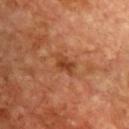biopsy status — total-body-photography surveillance lesion; no biopsy
site — the chest
patient — male, in their 60s
image — ~15 mm crop, total-body skin-cancer survey